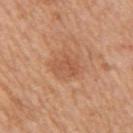follow-up = catalogued during a skin exam; not biopsied
anatomic site = the right upper arm
TBP lesion metrics = a mean CIELAB color near L≈55 a*≈25 b*≈35, a lesion–skin lightness drop of about 7, and a normalized lesion–skin contrast near 5; a border-irregularity rating of about 4.5/10, a color-variation rating of about 3.5/10, and peripheral color asymmetry of about 1.5
subject = female, aged 53 to 57
lesion diameter = about 3 mm
image source = 15 mm crop, total-body photography
illumination = white-light